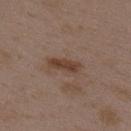Case summary:
- anatomic site: the upper back
- subject: female, aged 33 to 37
- automated metrics: a footprint of about 6 mm², an eccentricity of roughly 0.9, and a shape-asymmetry score of about 0.15 (0 = symmetric); a mean CIELAB color near L≈41 a*≈17 b*≈26, roughly 9 lightness units darker than nearby skin, and a normalized border contrast of about 8; a border-irregularity index near 2.5/10, a color-variation rating of about 2.5/10, and radial color variation of about 1; an automated nevus-likeness rating near 65 out of 100
- lighting: white-light illumination
- imaging modality: ~15 mm tile from a whole-body skin photo
- size: ≈4 mm